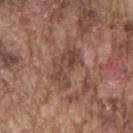Imaged during a routine full-body skin examination; the lesion was not biopsied and no histopathology is available. A male patient, aged approximately 75. Imaged with white-light lighting. Approximately 5 mm at its widest. Cropped from a total-body skin-imaging series; the visible field is about 15 mm. The lesion is on the mid back.